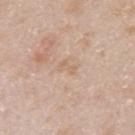biopsy status: imaged on a skin check; not biopsied | image: ~15 mm tile from a whole-body skin photo | location: the left upper arm | lighting: white-light | image-analysis metrics: a lesion-to-skin contrast of about 5 (normalized; higher = more distinct); a classifier nevus-likeness of about 0/100 and a lesion-detection confidence of about 100/100 | subject: male, approximately 75 years of age | size: about 2.5 mm.